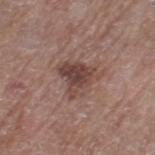The recorded lesion diameter is about 4.5 mm.
Located on the left thigh.
This image is a 15 mm lesion crop taken from a total-body photograph.
A female patient, in their 80s.
This is a white-light tile.